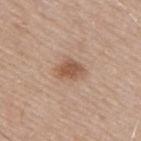Case summary:
– biopsy status: no biopsy performed (imaged during a skin exam)
– anatomic site: the upper back
– patient: male, roughly 65 years of age
– size: about 3.5 mm
– image: ~15 mm crop, total-body skin-cancer survey